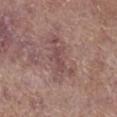Clinical impression:
Captured during whole-body skin photography for melanoma surveillance; the lesion was not biopsied.
Acquisition and patient details:
The lesion-visualizer software estimated a footprint of about 12 mm² and two-axis asymmetry of about 0.35. It also reported a border-irregularity index near 6/10 and a peripheral color-asymmetry measure near 1. It also reported an automated nevus-likeness rating near 0 out of 100 and a detector confidence of about 90 out of 100 that the crop contains a lesion. Imaged with white-light lighting. The subject is a female in their mid- to late 70s. A close-up tile cropped from a whole-body skin photograph, about 15 mm across. Approximately 6 mm at its widest. On the right lower leg.